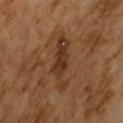biopsy_status: not biopsied; imaged during a skin examination
patient:
  sex: male
  age_approx: 65
image:
  source: total-body photography crop
  field_of_view_mm: 15
automated_metrics:
  area_mm2_approx: 11.0
  shape_asymmetry: 0.35
  cielab_L: 33
  cielab_a: 20
  cielab_b: 30
  nevus_likeness_0_100: 0
  lesion_detection_confidence_0_100: 100